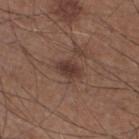notes: imaged on a skin check; not biopsied | imaging modality: ~15 mm crop, total-body skin-cancer survey | subject: male, about 60 years old | tile lighting: white-light | diameter: about 3 mm | automated lesion analysis: a footprint of about 4.5 mm², an outline eccentricity of about 0.8 (0 = round, 1 = elongated), and a symmetry-axis asymmetry near 0.15; a border-irregularity rating of about 1.5/10 and a within-lesion color-variation index near 2/10; an automated nevus-likeness rating near 85 out of 100 | site: the left lower leg.